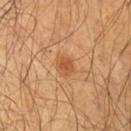Case summary:
• image — ~15 mm crop, total-body skin-cancer survey
• location — the chest
• lighting — cross-polarized
• subject — male, aged around 60